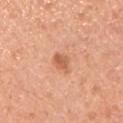Q: Was a biopsy performed?
A: no biopsy performed (imaged during a skin exam)
Q: Lesion size?
A: about 2.5 mm
Q: Patient demographics?
A: male, roughly 45 years of age
Q: Where on the body is the lesion?
A: the front of the torso
Q: How was the tile lit?
A: white-light
Q: Automated lesion metrics?
A: an eccentricity of roughly 0.65 and a shape-asymmetry score of about 0.25 (0 = symmetric); a border-irregularity index near 2/10, internal color variation of about 2 on a 0–10 scale, and a peripheral color-asymmetry measure near 0.5; a classifier nevus-likeness of about 65/100 and a detector confidence of about 100 out of 100 that the crop contains a lesion
Q: What kind of image is this?
A: 15 mm crop, total-body photography